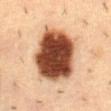biopsy status = imaged on a skin check; not biopsied
automated lesion analysis = an automated nevus-likeness rating near 95 out of 100 and a lesion-detection confidence of about 100/100
illumination = cross-polarized
patient = male, aged approximately 55
lesion diameter = ≈7 mm
site = the back
acquisition = 15 mm crop, total-body photography